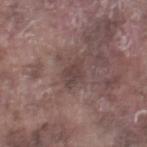Findings:
- subject: male, aged 73–77
- diameter: about 3 mm
- site: the leg
- image: ~15 mm crop, total-body skin-cancer survey
- lighting: white-light illumination
- automated metrics: a lesion area of about 5 mm² and a shape-asymmetry score of about 0.2 (0 = symmetric); a lesion color around L≈41 a*≈16 b*≈18 in CIELAB, a lesion–skin lightness drop of about 7, and a normalized lesion–skin contrast near 6; border irregularity of about 2 on a 0–10 scale, a color-variation rating of about 1.5/10, and radial color variation of about 0.5; an automated nevus-likeness rating near 0 out of 100 and a detector confidence of about 90 out of 100 that the crop contains a lesion